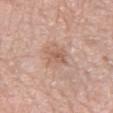| feature | finding |
|---|---|
| workup | imaged on a skin check; not biopsied |
| patient | female, aged 38–42 |
| lighting | white-light |
| acquisition | 15 mm crop, total-body photography |
| image-analysis metrics | a footprint of about 5 mm², an eccentricity of roughly 0.65, and a shape-asymmetry score of about 0.25 (0 = symmetric); an average lesion color of about L≈59 a*≈20 b*≈28 (CIELAB), about 8 CIELAB-L* units darker than the surrounding skin, and a normalized border contrast of about 6; a color-variation rating of about 2/10 |
| location | the left forearm |